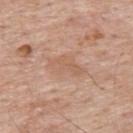No biopsy was performed on this lesion — it was imaged during a full skin examination and was not determined to be concerning.
A 15 mm close-up extracted from a 3D total-body photography capture.
The recorded lesion diameter is about 4.5 mm.
This is a white-light tile.
Automated tile analysis of the lesion measured a border-irregularity index near 4/10, a within-lesion color-variation index near 2/10, and a peripheral color-asymmetry measure near 0.5. It also reported an automated nevus-likeness rating near 0 out of 100 and a lesion-detection confidence of about 100/100.
On the upper back.
A male subject, in their 60s.